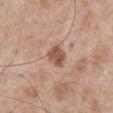Clinical impression:
Recorded during total-body skin imaging; not selected for excision or biopsy.
Background:
A male subject, approximately 50 years of age. Imaged with white-light lighting. A close-up tile cropped from a whole-body skin photograph, about 15 mm across. The lesion is on the mid back.